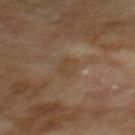Captured during whole-body skin photography for melanoma surveillance; the lesion was not biopsied. The lesion is located on the mid back. A 15 mm crop from a total-body photograph taken for skin-cancer surveillance. A male patient in their 70s.